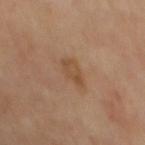Findings:
* notes · total-body-photography surveillance lesion; no biopsy
* site · the back
* acquisition · total-body-photography crop, ~15 mm field of view
* illumination · cross-polarized